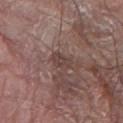follow-up: no biopsy performed (imaged during a skin exam); lighting: white-light illumination; patient: male, in their 80s; diameter: ~3 mm (longest diameter); location: the left arm; imaging modality: ~15 mm tile from a whole-body skin photo.